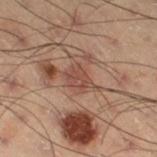Assessment:
Part of a total-body skin-imaging series; this lesion was reviewed on a skin check and was not flagged for biopsy.
Image and clinical context:
A 15 mm close-up extracted from a 3D total-body photography capture. An algorithmic analysis of the crop reported about 7 CIELAB-L* units darker than the surrounding skin. The lesion's longest dimension is about 3 mm. A male patient, about 55 years old. Located on the right thigh. This is a cross-polarized tile.